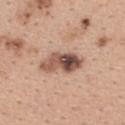An algorithmic analysis of the crop reported a lesion area of about 11 mm² and an outline eccentricity of about 0.9 (0 = round, 1 = elongated). The software also gave a lesion color around L≈53 a*≈20 b*≈27 in CIELAB, roughly 16 lightness units darker than nearby skin, and a normalized border contrast of about 10.5. The software also gave a border-irregularity index near 3/10 and radial color variation of about 4.5. A roughly 15 mm field-of-view crop from a total-body skin photograph. A female patient aged 38–42. The lesion is on the upper back. Longest diameter approximately 5.5 mm.